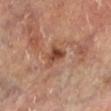Part of a total-body skin-imaging series; this lesion was reviewed on a skin check and was not flagged for biopsy. The subject is a male roughly 70 years of age. Approximately 3 mm at its widest. From the left lower leg. This image is a 15 mm lesion crop taken from a total-body photograph.